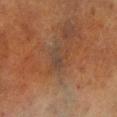Part of a total-body skin-imaging series; this lesion was reviewed on a skin check and was not flagged for biopsy.